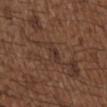| key | value |
|---|---|
| biopsy status | imaged on a skin check; not biopsied |
| site | the upper back |
| automated lesion analysis | an average lesion color of about L≈33 a*≈17 b*≈23 (CIELAB), about 5 CIELAB-L* units darker than the surrounding skin, and a lesion-to-skin contrast of about 5.5 (normalized; higher = more distinct); an automated nevus-likeness rating near 0 out of 100 and lesion-presence confidence of about 55/100 |
| patient | male, approximately 50 years of age |
| tile lighting | white-light |
| image source | ~15 mm tile from a whole-body skin photo |